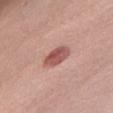follow-up: imaged on a skin check; not biopsied | automated lesion analysis: a footprint of about 6.5 mm², an outline eccentricity of about 0.8 (0 = round, 1 = elongated), and a shape-asymmetry score of about 0.15 (0 = symmetric); a border-irregularity index near 1.5/10, a within-lesion color-variation index near 3.5/10, and peripheral color asymmetry of about 1 | image source: total-body-photography crop, ~15 mm field of view | lesion diameter: ~3.5 mm (longest diameter) | body site: the right thigh | tile lighting: white-light | subject: female, in their 40s.